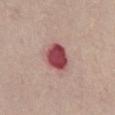Captured during whole-body skin photography for melanoma surveillance; the lesion was not biopsied. A female subject aged around 65. A 15 mm close-up tile from a total-body photography series done for melanoma screening. The lesion is on the abdomen.